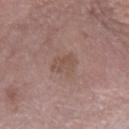This lesion was catalogued during total-body skin photography and was not selected for biopsy. Longest diameter approximately 3.5 mm. From the left forearm. A close-up tile cropped from a whole-body skin photograph, about 15 mm across. The tile uses white-light illumination. The patient is a male aged around 75.